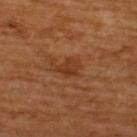Part of a total-body skin-imaging series; this lesion was reviewed on a skin check and was not flagged for biopsy. Captured under cross-polarized illumination. An algorithmic analysis of the crop reported a lesion area of about 5 mm². And it measured an average lesion color of about L≈35 a*≈23 b*≈34 (CIELAB), about 7 CIELAB-L* units darker than the surrounding skin, and a normalized border contrast of about 6.5. A 15 mm close-up tile from a total-body photography series done for melanoma screening. A male patient aged approximately 65. On the upper back. Longest diameter approximately 3 mm.